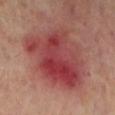Q: Was a biopsy performed?
A: no biopsy performed (imaged during a skin exam)
Q: How was the tile lit?
A: cross-polarized
Q: What are the patient's age and sex?
A: female, in their mid- to late 50s
Q: Automated lesion metrics?
A: a shape eccentricity near 0.65; a lesion-detection confidence of about 100/100
Q: Lesion size?
A: about 8.5 mm
Q: How was this image acquired?
A: total-body-photography crop, ~15 mm field of view
Q: Where on the body is the lesion?
A: the left lower leg